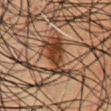Case summary:
• follow-up · catalogued during a skin exam; not biopsied
• site · the chest
• diameter · ≈6.5 mm
• lighting · cross-polarized illumination
• subject · male, aged around 50
• acquisition · 15 mm crop, total-body photography